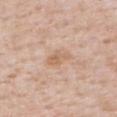Q: Was this lesion biopsied?
A: no biopsy performed (imaged during a skin exam)
Q: How was this image acquired?
A: total-body-photography crop, ~15 mm field of view
Q: Who is the patient?
A: male, about 50 years old
Q: Lesion location?
A: the upper back